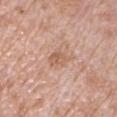This lesion was catalogued during total-body skin photography and was not selected for biopsy. This image is a 15 mm lesion crop taken from a total-body photograph. Approximately 2.5 mm at its widest. The lesion is on the right upper arm. A male subject in their 70s. Captured under white-light illumination. Automated image analysis of the tile measured an eccentricity of roughly 0.7 and a symmetry-axis asymmetry near 0.25. And it measured a border-irregularity index near 2.5/10, internal color variation of about 2.5 on a 0–10 scale, and peripheral color asymmetry of about 1.